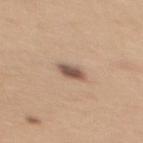| key | value |
|---|---|
| notes | catalogued during a skin exam; not biopsied |
| patient | female, aged 28–32 |
| anatomic site | the upper back |
| image source | ~15 mm tile from a whole-body skin photo |
| diameter | ≈2.5 mm |